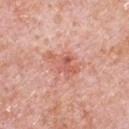Findings:
– workup — imaged on a skin check; not biopsied
– imaging modality — ~15 mm crop, total-body skin-cancer survey
– lesion size — about 4 mm
– illumination — white-light
– patient — male, about 80 years old
– body site — the chest